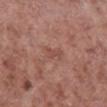Part of a total-body skin-imaging series; this lesion was reviewed on a skin check and was not flagged for biopsy. The lesion's longest dimension is about 2.5 mm. This is a white-light tile. The lesion is located on the right lower leg. A male subject, aged 53 to 57. A 15 mm close-up extracted from a 3D total-body photography capture.